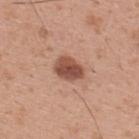Q: Was a biopsy performed?
A: imaged on a skin check; not biopsied
Q: How was the tile lit?
A: white-light
Q: Lesion size?
A: ≈3.5 mm
Q: What is the anatomic site?
A: the upper back
Q: What did automated image analysis measure?
A: an automated nevus-likeness rating near 95 out of 100
Q: What kind of image is this?
A: total-body-photography crop, ~15 mm field of view
Q: What are the patient's age and sex?
A: male, aged 28 to 32